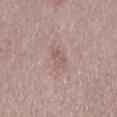Clinical summary:
A region of skin cropped from a whole-body photographic capture, roughly 15 mm wide. The tile uses white-light illumination. Approximately 3 mm at its widest. Automated tile analysis of the lesion measured an area of roughly 4 mm², an eccentricity of roughly 0.8, and a symmetry-axis asymmetry near 0.35. The software also gave roughly 7 lightness units darker than nearby skin and a lesion-to-skin contrast of about 5 (normalized; higher = more distinct). And it measured a color-variation rating of about 2/10 and peripheral color asymmetry of about 1. Located on the mid back. A male patient aged approximately 70.